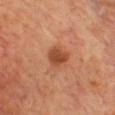Captured during whole-body skin photography for melanoma surveillance; the lesion was not biopsied. An algorithmic analysis of the crop reported a mean CIELAB color near L≈46 a*≈26 b*≈34, about 11 CIELAB-L* units darker than the surrounding skin, and a normalized lesion–skin contrast near 8.5. And it measured a border-irregularity index near 2.5/10, a within-lesion color-variation index near 2.5/10, and peripheral color asymmetry of about 0.5. And it measured a classifier nevus-likeness of about 90/100 and lesion-presence confidence of about 100/100. The lesion is on the chest. Approximately 3 mm at its widest. Captured under cross-polarized illumination. A male patient, about 70 years old. A roughly 15 mm field-of-view crop from a total-body skin photograph.